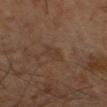Recorded during total-body skin imaging; not selected for excision or biopsy. The subject is a male aged around 60. On the right arm. An algorithmic analysis of the crop reported an average lesion color of about L≈34 a*≈15 b*≈25 (CIELAB), about 5 CIELAB-L* units darker than the surrounding skin, and a lesion-to-skin contrast of about 4.5 (normalized; higher = more distinct). The software also gave an automated nevus-likeness rating near 0 out of 100 and a lesion-detection confidence of about 100/100. A roughly 15 mm field-of-view crop from a total-body skin photograph.